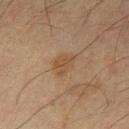{
  "biopsy_status": "not biopsied; imaged during a skin examination",
  "lighting": "cross-polarized",
  "automated_metrics": {
    "shape_asymmetry": 0.3,
    "cielab_L": 38,
    "cielab_a": 14,
    "cielab_b": 27,
    "vs_skin_darker_L": 6.0,
    "vs_skin_contrast_norm": 5.5,
    "color_variation_0_10": 1.5,
    "peripheral_color_asymmetry": 0.5
  },
  "image": {
    "source": "total-body photography crop",
    "field_of_view_mm": 15
  },
  "site": "leg",
  "lesion_size": {
    "long_diameter_mm_approx": 3.0
  },
  "patient": {
    "sex": "male",
    "age_approx": 65
  }
}